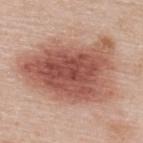Clinical impression: No biopsy was performed on this lesion — it was imaged during a full skin examination and was not determined to be concerning. Clinical summary: A female patient about 50 years old. On the upper back. A 15 mm close-up extracted from a 3D total-body photography capture.